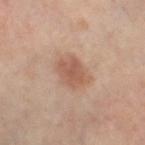* subject: female, aged around 50
* location: the left thigh
* image source: 15 mm crop, total-body photography
* tile lighting: cross-polarized
* lesion diameter: ~4 mm (longest diameter)